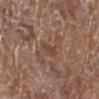Recorded during total-body skin imaging; not selected for excision or biopsy.
From the left lower leg.
A lesion tile, about 15 mm wide, cut from a 3D total-body photograph.
A female patient, approximately 80 years of age.